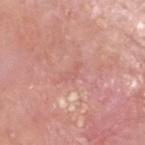{"patient": {"sex": "male", "age_approx": 60}, "image": {"source": "total-body photography crop", "field_of_view_mm": 15}, "site": "head or neck", "diagnosis": {"histopathology": "squamous cell carcinoma in situ", "malignancy": "malignant", "taxonomic_path": ["Malignant", "Malignant epidermal proliferations", "Squamous cell carcinoma in situ"]}}